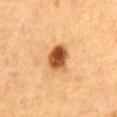Imaged during a routine full-body skin examination; the lesion was not biopsied and no histopathology is available.